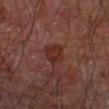notes: no biopsy performed (imaged during a skin exam) | imaging modality: ~15 mm tile from a whole-body skin photo | subject: male, aged around 60 | body site: the right forearm.